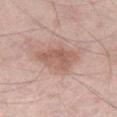| feature | finding |
|---|---|
| workup | no biopsy performed (imaged during a skin exam) |
| imaging modality | 15 mm crop, total-body photography |
| body site | the leg |
| patient | male, about 55 years old |